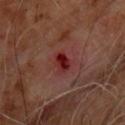This lesion was catalogued during total-body skin photography and was not selected for biopsy. A male subject, aged 58 to 62. The tile uses cross-polarized illumination. A 15 mm crop from a total-body photograph taken for skin-cancer surveillance. The lesion is located on the chest. The lesion's longest dimension is about 2.5 mm.